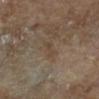Notes:
- biopsy status — total-body-photography surveillance lesion; no biopsy
- anatomic site — the right lower leg
- patient — male, roughly 85 years of age or older
- diameter — ≈3 mm
- imaging modality — total-body-photography crop, ~15 mm field of view
- lighting — cross-polarized